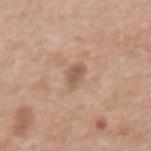workup: no biopsy performed (imaged during a skin exam) | automated lesion analysis: a footprint of about 4 mm², an eccentricity of roughly 0.85, and a symmetry-axis asymmetry near 0.3; internal color variation of about 1.5 on a 0–10 scale and a peripheral color-asymmetry measure near 0.5 | lesion diameter: about 3 mm | imaging modality: ~15 mm tile from a whole-body skin photo | patient: female, in their mid-70s | anatomic site: the abdomen.